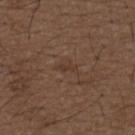Impression:
Imaged during a routine full-body skin examination; the lesion was not biopsied and no histopathology is available.
Clinical summary:
On the back. Automated image analysis of the tile measured an average lesion color of about L≈36 a*≈16 b*≈25 (CIELAB) and a normalized border contrast of about 5. The software also gave internal color variation of about 0 on a 0–10 scale. And it measured an automated nevus-likeness rating near 0 out of 100 and a detector confidence of about 95 out of 100 that the crop contains a lesion. The subject is a male in their 50s. Captured under white-light illumination. A region of skin cropped from a whole-body photographic capture, roughly 15 mm wide.